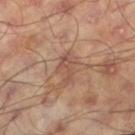Impression:
The lesion was photographed on a routine skin check and not biopsied; there is no pathology result.
Acquisition and patient details:
Imaged with cross-polarized lighting. On the left thigh. A male patient aged 58–62. This image is a 15 mm lesion crop taken from a total-body photograph.